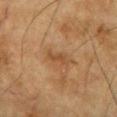This lesion was catalogued during total-body skin photography and was not selected for biopsy. The tile uses cross-polarized illumination. The lesion is located on the front of the torso. Automated image analysis of the tile measured a footprint of about 3 mm² and a shape eccentricity near 0.9. The analysis additionally found a lesion color around L≈42 a*≈18 b*≈32 in CIELAB, about 7 CIELAB-L* units darker than the surrounding skin, and a normalized border contrast of about 6. The analysis additionally found an automated nevus-likeness rating near 0 out of 100. The recorded lesion diameter is about 3 mm. A male subject about 85 years old or older. Cropped from a whole-body photographic skin survey; the tile spans about 15 mm.